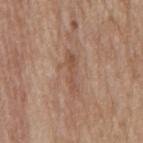Recorded during total-body skin imaging; not selected for excision or biopsy. A close-up tile cropped from a whole-body skin photograph, about 15 mm across. Located on the back. A female patient, aged 63–67. The tile uses white-light illumination. Automated image analysis of the tile measured a footprint of about 5.5 mm² and a symmetry-axis asymmetry near 0.4. And it measured a mean CIELAB color near L≈52 a*≈19 b*≈28, roughly 8 lightness units darker than nearby skin, and a lesion-to-skin contrast of about 6 (normalized; higher = more distinct). The analysis additionally found border irregularity of about 7.5 on a 0–10 scale, a within-lesion color-variation index near 1.5/10, and peripheral color asymmetry of about 0.5. And it measured a nevus-likeness score of about 0/100 and a detector confidence of about 90 out of 100 that the crop contains a lesion. The lesion's longest dimension is about 5 mm.